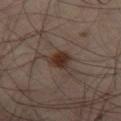Imaged during a routine full-body skin examination; the lesion was not biopsied and no histopathology is available.
An algorithmic analysis of the crop reported a classifier nevus-likeness of about 100/100 and lesion-presence confidence of about 100/100.
Located on the lower back.
A male subject, in their 50s.
A roughly 15 mm field-of-view crop from a total-body skin photograph.
Approximately 3.5 mm at its widest.
Captured under cross-polarized illumination.